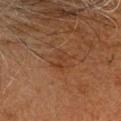  biopsy_status: not biopsied; imaged during a skin examination
  patient:
    sex: male
    age_approx: 65
  image:
    source: total-body photography crop
    field_of_view_mm: 15
  site: head or neck
  lesion_size:
    long_diameter_mm_approx: 3.0
  lighting: cross-polarized